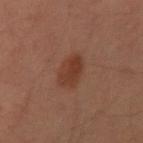{
  "biopsy_status": "not biopsied; imaged during a skin examination",
  "patient": {
    "sex": "male",
    "age_approx": 35
  },
  "image": {
    "source": "total-body photography crop",
    "field_of_view_mm": 15
  },
  "automated_metrics": {
    "cielab_L": 33,
    "cielab_a": 21,
    "cielab_b": 26,
    "vs_skin_darker_L": 7.0,
    "vs_skin_contrast_norm": 7.5,
    "border_irregularity_0_10": 2.5,
    "color_variation_0_10": 2.5
  },
  "site": "right upper arm",
  "lighting": "cross-polarized",
  "lesion_size": {
    "long_diameter_mm_approx": 3.5
  }
}